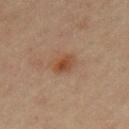  automated_metrics:
    area_mm2_approx: 4.0
    eccentricity: 0.75
  patient:
    sex: female
    age_approx: 45
  lighting: cross-polarized
  lesion_size:
    long_diameter_mm_approx: 2.5
  site: arm
  image:
    source: total-body photography crop
    field_of_view_mm: 15